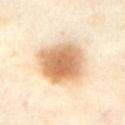{"biopsy_status": "not biopsied; imaged during a skin examination", "site": "abdomen", "image": {"source": "total-body photography crop", "field_of_view_mm": 15}, "patient": {"sex": "female", "age_approx": 55}, "lighting": "cross-polarized", "automated_metrics": {"eccentricity": 0.45, "shape_asymmetry": 0.15, "cielab_L": 72, "cielab_a": 20, "cielab_b": 38, "vs_skin_darker_L": 17.0}}